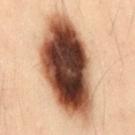biopsy status = imaged on a skin check; not biopsied
imaging modality = ~15 mm crop, total-body skin-cancer survey
tile lighting = cross-polarized illumination
automated lesion analysis = an area of roughly 55 mm² and two-axis asymmetry of about 0.15
subject = male, in their 50s
lesion diameter = ~11 mm (longest diameter)
location = the lower back